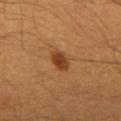The lesion was tiled from a total-body skin photograph and was not biopsied. Cropped from a whole-body photographic skin survey; the tile spans about 15 mm. A male subject, aged 58–62. On the mid back.